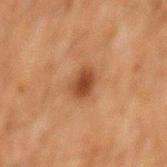Notes:
– notes — no biopsy performed (imaged during a skin exam)
– lesion diameter — about 3 mm
– subject — male, aged around 60
– imaging modality — 15 mm crop, total-body photography
– TBP lesion metrics — a footprint of about 5.5 mm², a shape eccentricity near 0.65, and a shape-asymmetry score of about 0.25 (0 = symmetric); a lesion color around L≈35 a*≈20 b*≈29 in CIELAB, roughly 10 lightness units darker than nearby skin, and a normalized lesion–skin contrast near 9; a classifier nevus-likeness of about 95/100 and lesion-presence confidence of about 100/100
– site — the mid back